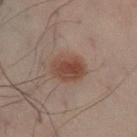Q: Was this lesion biopsied?
A: imaged on a skin check; not biopsied
Q: What are the patient's age and sex?
A: male, aged 48 to 52
Q: What is the anatomic site?
A: the left leg
Q: What kind of image is this?
A: ~15 mm tile from a whole-body skin photo